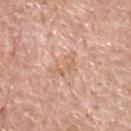location — the upper back | image source — ~15 mm tile from a whole-body skin photo | patient — male, approximately 70 years of age.